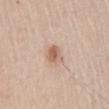The lesion was tiled from a total-body skin photograph and was not biopsied.
Longest diameter approximately 2.5 mm.
A 15 mm close-up extracted from a 3D total-body photography capture.
From the front of the torso.
An algorithmic analysis of the crop reported a lesion color around L≈61 a*≈19 b*≈30 in CIELAB and a normalized border contrast of about 7. The analysis additionally found a detector confidence of about 100 out of 100 that the crop contains a lesion.
A male subject, aged 63–67.